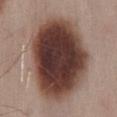Q: Is there a histopathology result?
A: total-body-photography surveillance lesion; no biopsy
Q: What is the anatomic site?
A: the mid back
Q: Who is the patient?
A: male, approximately 55 years of age
Q: What kind of image is this?
A: ~15 mm crop, total-body skin-cancer survey